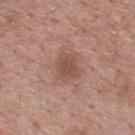biopsy status = total-body-photography surveillance lesion; no biopsy | diameter = about 3 mm | body site = the back | image source = total-body-photography crop, ~15 mm field of view | subject = male, aged 68–72.